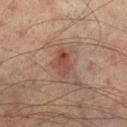– follow-up · imaged on a skin check; not biopsied
– lesion diameter · ~3.5 mm (longest diameter)
– lighting · cross-polarized
– imaging modality · ~15 mm crop, total-body skin-cancer survey
– patient · male, approximately 75 years of age
– anatomic site · the right lower leg
– automated metrics · a mean CIELAB color near L≈38 a*≈20 b*≈23, about 8 CIELAB-L* units darker than the surrounding skin, and a normalized lesion–skin contrast near 7; border irregularity of about 2 on a 0–10 scale; a nevus-likeness score of about 30/100 and a lesion-detection confidence of about 100/100